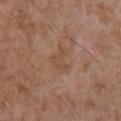Impression: Part of a total-body skin-imaging series; this lesion was reviewed on a skin check and was not flagged for biopsy. Image and clinical context: Longest diameter approximately 3.5 mm. An algorithmic analysis of the crop reported a lesion area of about 5.5 mm², an eccentricity of roughly 0.75, and a shape-asymmetry score of about 0.5 (0 = symmetric). The analysis additionally found roughly 5 lightness units darker than nearby skin and a lesion-to-skin contrast of about 5 (normalized; higher = more distinct). The analysis additionally found a border-irregularity rating of about 5/10, internal color variation of about 1 on a 0–10 scale, and peripheral color asymmetry of about 0.5. A male subject aged around 60. A lesion tile, about 15 mm wide, cut from a 3D total-body photograph. The tile uses white-light illumination. Located on the back.